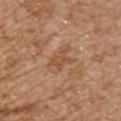Assessment: The lesion was tiled from a total-body skin photograph and was not biopsied. Background: The tile uses white-light illumination. Automated tile analysis of the lesion measured a lesion area of about 4 mm², an outline eccentricity of about 0.65 (0 = round, 1 = elongated), and two-axis asymmetry of about 0.4. The analysis additionally found a mean CIELAB color near L≈51 a*≈22 b*≈34, about 7 CIELAB-L* units darker than the surrounding skin, and a normalized lesion–skin contrast near 5.5. And it measured border irregularity of about 4.5 on a 0–10 scale, internal color variation of about 1.5 on a 0–10 scale, and a peripheral color-asymmetry measure near 0.5. This image is a 15 mm lesion crop taken from a total-body photograph. A female patient, approximately 30 years of age. The lesion is located on the chest. Approximately 3 mm at its widest.